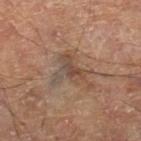Assessment: No biopsy was performed on this lesion — it was imaged during a full skin examination and was not determined to be concerning. Background: This image is a 15 mm lesion crop taken from a total-body photograph. The lesion is on the right thigh.